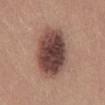No biopsy was performed on this lesion — it was imaged during a full skin examination and was not determined to be concerning. This image is a 15 mm lesion crop taken from a total-body photograph. On the front of the torso. The patient is a male aged approximately 25.